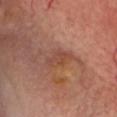The lesion is located on the head or neck. The subject is a male approximately 65 years of age. Cropped from a total-body skin-imaging series; the visible field is about 15 mm.